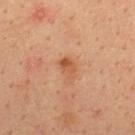A male subject, in their mid-30s.
A 15 mm close-up extracted from a 3D total-body photography capture.
Captured under cross-polarized illumination.
On the upper back.